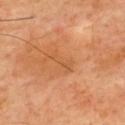Captured during whole-body skin photography for melanoma surveillance; the lesion was not biopsied. Captured under cross-polarized illumination. This image is a 15 mm lesion crop taken from a total-body photograph. A male subject, approximately 55 years of age. From the upper back. An algorithmic analysis of the crop reported a lesion area of about 3 mm², an outline eccentricity of about 0.95 (0 = round, 1 = elongated), and a shape-asymmetry score of about 0.4 (0 = symmetric). The software also gave an average lesion color of about L≈55 a*≈26 b*≈41 (CIELAB), about 6 CIELAB-L* units darker than the surrounding skin, and a normalized border contrast of about 4. The software also gave a border-irregularity rating of about 5.5/10, a color-variation rating of about 0/10, and peripheral color asymmetry of about 0. The analysis additionally found a nevus-likeness score of about 0/100 and a lesion-detection confidence of about 100/100.